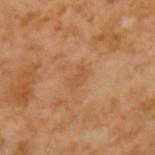The lesion was tiled from a total-body skin photograph and was not biopsied. Cropped from a whole-body photographic skin survey; the tile spans about 15 mm. A male subject aged 63–67.